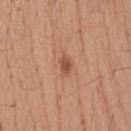Part of a total-body skin-imaging series; this lesion was reviewed on a skin check and was not flagged for biopsy. About 2.5 mm across. The lesion is located on the head or neck. A roughly 15 mm field-of-view crop from a total-body skin photograph. An algorithmic analysis of the crop reported a footprint of about 3 mm², a shape eccentricity near 0.8, and two-axis asymmetry of about 0.25. The software also gave a mean CIELAB color near L≈52 a*≈24 b*≈33 and a lesion–skin lightness drop of about 11. The analysis additionally found a border-irregularity rating of about 2.5/10, a color-variation rating of about 1.5/10, and peripheral color asymmetry of about 0.5. A male patient, aged around 20.